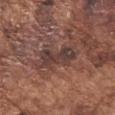Assessment: The lesion was tiled from a total-body skin photograph and was not biopsied. Context: A 15 mm crop from a total-body photograph taken for skin-cancer surveillance. A male subject, aged 73–77. This is a white-light tile. The lesion's longest dimension is about 5 mm. Located on the left upper arm.